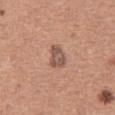Part of a total-body skin-imaging series; this lesion was reviewed on a skin check and was not flagged for biopsy. A female subject in their 50s. A roughly 15 mm field-of-view crop from a total-body skin photograph. On the right thigh. The total-body-photography lesion software estimated a lesion area of about 5.5 mm², an outline eccentricity of about 0.65 (0 = round, 1 = elongated), and a symmetry-axis asymmetry near 0.3. It also reported a lesion color around L≈53 a*≈20 b*≈26 in CIELAB, about 11 CIELAB-L* units darker than the surrounding skin, and a normalized border contrast of about 7.5. It also reported a color-variation rating of about 3.5/10. The lesion's longest dimension is about 3 mm.